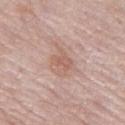<record>
<biopsy_status>not biopsied; imaged during a skin examination</biopsy_status>
<site>left thigh</site>
<image>
  <source>total-body photography crop</source>
  <field_of_view_mm>15</field_of_view_mm>
</image>
<automated_metrics>
  <border_irregularity_0_10>4.5</border_irregularity_0_10>
  <color_variation_0_10>3.0</color_variation_0_10>
  <peripheral_color_asymmetry>1.0</peripheral_color_asymmetry>
  <nevus_likeness_0_100>0</nevus_likeness_0_100>
</automated_metrics>
<patient>
  <sex>female</sex>
  <age_approx>60</age_approx>
</patient>
</record>